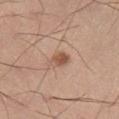<record>
  <biopsy_status>not biopsied; imaged during a skin examination</biopsy_status>
  <lesion_size>
    <long_diameter_mm_approx>2.5</long_diameter_mm_approx>
  </lesion_size>
  <image>
    <source>total-body photography crop</source>
    <field_of_view_mm>15</field_of_view_mm>
  </image>
  <patient>
    <sex>male</sex>
    <age_approx>30</age_approx>
  </patient>
  <lighting>white-light</lighting>
  <automated_metrics>
    <cielab_L>54</cielab_L>
    <cielab_a>21</cielab_a>
    <cielab_b>30</cielab_b>
    <vs_skin_darker_L>11.0</vs_skin_darker_L>
    <peripheral_color_asymmetry>1.5</peripheral_color_asymmetry>
  </automated_metrics>
  <site>right lower leg</site>
</record>